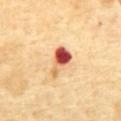<record>
  <biopsy_status>not biopsied; imaged during a skin examination</biopsy_status>
  <lighting>cross-polarized</lighting>
  <patient>
    <sex>male</sex>
    <age_approx>65</age_approx>
  </patient>
  <site>abdomen</site>
  <image>
    <source>total-body photography crop</source>
    <field_of_view_mm>15</field_of_view_mm>
  </image>
  <lesion_size>
    <long_diameter_mm_approx>4.0</long_diameter_mm_approx>
  </lesion_size>
</record>